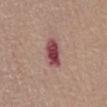| field | value |
|---|---|
| notes | catalogued during a skin exam; not biopsied |
| TBP lesion metrics | a border-irregularity index near 2/10, a color-variation rating of about 4/10, and radial color variation of about 1.5 |
| site | the abdomen |
| patient | female, aged approximately 65 |
| image | ~15 mm crop, total-body skin-cancer survey |
| tile lighting | white-light |